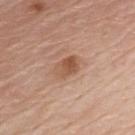The lesion was photographed on a routine skin check and not biopsied; there is no pathology result. A roughly 15 mm field-of-view crop from a total-body skin photograph. The lesion-visualizer software estimated a classifier nevus-likeness of about 60/100 and a lesion-detection confidence of about 100/100. The lesion is on the chest. The tile uses white-light illumination. A male patient, about 80 years old. Approximately 3.5 mm at its widest.